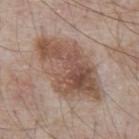<case>
  <image>
    <source>total-body photography crop</source>
    <field_of_view_mm>15</field_of_view_mm>
  </image>
  <site>abdomen</site>
  <lesion_size>
    <long_diameter_mm_approx>9.0</long_diameter_mm_approx>
  </lesion_size>
  <automated_metrics>
    <cielab_L>52</cielab_L>
    <cielab_a>17</cielab_a>
    <cielab_b>26</cielab_b>
    <vs_skin_contrast_norm>8.0</vs_skin_contrast_norm>
    <border_irregularity_0_10>2.5</border_irregularity_0_10>
    <color_variation_0_10>7.0</color_variation_0_10>
    <peripheral_color_asymmetry>2.5</peripheral_color_asymmetry>
    <nevus_likeness_0_100>25</nevus_likeness_0_100>
    <lesion_detection_confidence_0_100>100</lesion_detection_confidence_0_100>
  </automated_metrics>
  <patient>
    <sex>male</sex>
    <age_approx>65</age_approx>
  </patient>
</case>